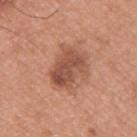Assessment: Captured during whole-body skin photography for melanoma surveillance; the lesion was not biopsied.